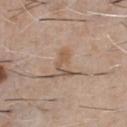Impression:
Captured during whole-body skin photography for melanoma surveillance; the lesion was not biopsied.
Clinical summary:
A male subject roughly 60 years of age. About 3.5 mm across. The lesion is on the chest. A 15 mm crop from a total-body photograph taken for skin-cancer surveillance.